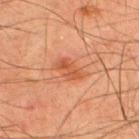<lesion>
  <patient>
    <sex>male</sex>
    <age_approx>45</age_approx>
  </patient>
  <automated_metrics>
    <cielab_L>45</cielab_L>
    <cielab_a>26</cielab_a>
    <cielab_b>33</cielab_b>
    <vs_skin_darker_L>8.0</vs_skin_darker_L>
    <border_irregularity_0_10>3.0</border_irregularity_0_10>
    <color_variation_0_10>3.0</color_variation_0_10>
    <peripheral_color_asymmetry>1.0</peripheral_color_asymmetry>
    <nevus_likeness_0_100>15</nevus_likeness_0_100>
    <lesion_detection_confidence_0_100>100</lesion_detection_confidence_0_100>
  </automated_metrics>
  <lesion_size>
    <long_diameter_mm_approx>3.5</long_diameter_mm_approx>
  </lesion_size>
  <image>
    <source>total-body photography crop</source>
    <field_of_view_mm>15</field_of_view_mm>
  </image>
  <site>upper back</site>
</lesion>